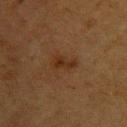Part of a total-body skin-imaging series; this lesion was reviewed on a skin check and was not flagged for biopsy. The patient is a female aged around 40. A lesion tile, about 15 mm wide, cut from a 3D total-body photograph. From the left upper arm. This is a cross-polarized tile. Measured at roughly 3.5 mm in maximum diameter. Automated tile analysis of the lesion measured a footprint of about 4.5 mm² and an eccentricity of roughly 0.85. The software also gave an average lesion color of about L≈24 a*≈16 b*≈26 (CIELAB), roughly 6 lightness units darker than nearby skin, and a normalized border contrast of about 7. It also reported lesion-presence confidence of about 100/100.